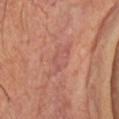<lesion>
<biopsy_status>not biopsied; imaged during a skin examination</biopsy_status>
<image>
  <source>total-body photography crop</source>
  <field_of_view_mm>15</field_of_view_mm>
</image>
<site>head or neck</site>
<lesion_size>
  <long_diameter_mm_approx>4.5</long_diameter_mm_approx>
</lesion_size>
<automated_metrics>
  <border_irregularity_0_10>6.0</border_irregularity_0_10>
  <color_variation_0_10>2.0</color_variation_0_10>
  <peripheral_color_asymmetry>0.5</peripheral_color_asymmetry>
  <nevus_likeness_0_100>0</nevus_likeness_0_100>
  <lesion_detection_confidence_0_100>70</lesion_detection_confidence_0_100>
</automated_metrics>
<lighting>cross-polarized</lighting>
<patient>
  <sex>male</sex>
  <age_approx>70</age_approx>
</patient>
</lesion>